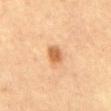Impression:
No biopsy was performed on this lesion — it was imaged during a full skin examination and was not determined to be concerning.
Context:
The tile uses cross-polarized illumination. A female subject, aged 53 to 57. Cropped from a whole-body photographic skin survey; the tile spans about 15 mm. About 3 mm across. On the abdomen.